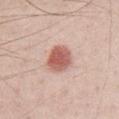body site=the front of the torso; acquisition=~15 mm tile from a whole-body skin photo; subject=male, in their 40s.